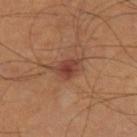Part of a total-body skin-imaging series; this lesion was reviewed on a skin check and was not flagged for biopsy.
A 15 mm close-up tile from a total-body photography series done for melanoma screening.
Imaged with cross-polarized lighting.
A male subject in their 50s.
The lesion is located on the right thigh.
Measured at roughly 3 mm in maximum diameter.
An algorithmic analysis of the crop reported an area of roughly 4.5 mm², a shape eccentricity near 0.65, and a symmetry-axis asymmetry near 0.25. It also reported a lesion color around L≈39 a*≈24 b*≈27 in CIELAB and about 9 CIELAB-L* units darker than the surrounding skin. The analysis additionally found border irregularity of about 2 on a 0–10 scale, a within-lesion color-variation index near 3/10, and a peripheral color-asymmetry measure near 1. And it measured an automated nevus-likeness rating near 90 out of 100.